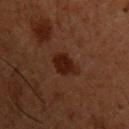The lesion was photographed on a routine skin check and not biopsied; there is no pathology result.
A lesion tile, about 15 mm wide, cut from a 3D total-body photograph.
A male patient about 60 years old.
An algorithmic analysis of the crop reported border irregularity of about 2.5 on a 0–10 scale, internal color variation of about 2 on a 0–10 scale, and a peripheral color-asymmetry measure near 0.5. And it measured a nevus-likeness score of about 95/100.
About 3.5 mm across.
Captured under cross-polarized illumination.
Located on the chest.